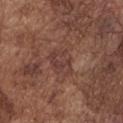Q: Was this lesion biopsied?
A: imaged on a skin check; not biopsied
Q: What is the anatomic site?
A: the chest
Q: How was this image acquired?
A: ~15 mm crop, total-body skin-cancer survey
Q: Patient demographics?
A: male, aged 73 to 77